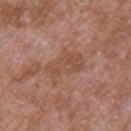follow-up: catalogued during a skin exam; not biopsied | acquisition: 15 mm crop, total-body photography | subject: male, about 45 years old | lesion size: ≈5 mm | image-analysis metrics: a mean CIELAB color near L≈50 a*≈21 b*≈28, roughly 6 lightness units darker than nearby skin, and a normalized border contrast of about 5; border irregularity of about 4 on a 0–10 scale and radial color variation of about 1 | illumination: white-light illumination | body site: the left upper arm.